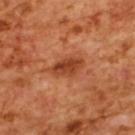size: about 4 mm | anatomic site: the upper back | image source: ~15 mm crop, total-body skin-cancer survey | illumination: cross-polarized | patient: female, in their mid-50s.